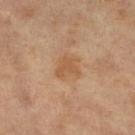Part of a total-body skin-imaging series; this lesion was reviewed on a skin check and was not flagged for biopsy.
Imaged with cross-polarized lighting.
The patient is a female aged 73 to 77.
The lesion is located on the leg.
The recorded lesion diameter is about 3 mm.
The total-body-photography lesion software estimated an area of roughly 6 mm², an eccentricity of roughly 0.25, and a symmetry-axis asymmetry near 0.3. And it measured a mean CIELAB color near L≈55 a*≈20 b*≈36, roughly 7 lightness units darker than nearby skin, and a normalized border contrast of about 6. The software also gave a classifier nevus-likeness of about 0/100 and a lesion-detection confidence of about 100/100.
A roughly 15 mm field-of-view crop from a total-body skin photograph.